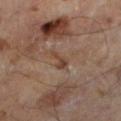Context:
A 15 mm crop from a total-body photograph taken for skin-cancer surveillance. A male subject, in their mid-60s. From the left lower leg. Imaged with cross-polarized lighting.